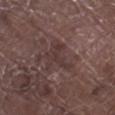workup = total-body-photography surveillance lesion; no biopsy
imaging modality = ~15 mm tile from a whole-body skin photo
location = the right lower leg
subject = male, roughly 80 years of age